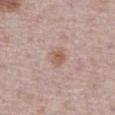Notes:
– biopsy status — total-body-photography surveillance lesion; no biopsy
– subject — male, aged 73–77
– automated lesion analysis — a footprint of about 5 mm², an outline eccentricity of about 0.6 (0 = round, 1 = elongated), and a shape-asymmetry score of about 0.3 (0 = symmetric); an average lesion color of about L≈58 a*≈19 b*≈26 (CIELAB) and about 8 CIELAB-L* units darker than the surrounding skin
– anatomic site — the chest
– size — ≈2.5 mm
– tile lighting — white-light
– image source — ~15 mm crop, total-body skin-cancer survey